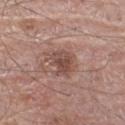Captured during whole-body skin photography for melanoma surveillance; the lesion was not biopsied.
About 3.5 mm across.
A male subject, roughly 75 years of age.
The tile uses white-light illumination.
A roughly 15 mm field-of-view crop from a total-body skin photograph.
The lesion is located on the left thigh.
The total-body-photography lesion software estimated a footprint of about 7 mm² and a symmetry-axis asymmetry near 0.25. The software also gave a lesion color around L≈48 a*≈20 b*≈24 in CIELAB, roughly 10 lightness units darker than nearby skin, and a normalized lesion–skin contrast near 7.5. The software also gave a nevus-likeness score of about 5/100.